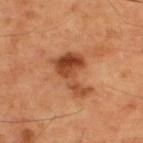{
  "lesion_size": {
    "long_diameter_mm_approx": 5.5
  },
  "image": {
    "source": "total-body photography crop",
    "field_of_view_mm": 15
  },
  "site": "back",
  "lighting": "cross-polarized",
  "patient": {
    "sex": "male",
    "age_approx": 60
  },
  "automated_metrics": {
    "nevus_likeness_0_100": 45,
    "lesion_detection_confidence_0_100": 100
  }
}